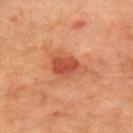This lesion was catalogued during total-body skin photography and was not selected for biopsy.
A roughly 15 mm field-of-view crop from a total-body skin photograph.
Automated tile analysis of the lesion measured an eccentricity of roughly 0.75 and a shape-asymmetry score of about 0.3 (0 = symmetric). The software also gave a mean CIELAB color near L≈52 a*≈30 b*≈36 and a lesion-to-skin contrast of about 7.5 (normalized; higher = more distinct). The software also gave border irregularity of about 3 on a 0–10 scale, internal color variation of about 5 on a 0–10 scale, and a peripheral color-asymmetry measure near 1.5. It also reported a classifier nevus-likeness of about 75/100 and a detector confidence of about 100 out of 100 that the crop contains a lesion.
On the right upper arm.
A male patient, roughly 65 years of age.